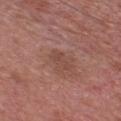{"biopsy_status": "not biopsied; imaged during a skin examination", "automated_metrics": {"vs_skin_darker_L": 6.0, "vs_skin_contrast_norm": 5.0, "border_irregularity_0_10": 5.0, "color_variation_0_10": 1.0, "peripheral_color_asymmetry": 0.0}, "lesion_size": {"long_diameter_mm_approx": 3.0}, "image": {"source": "total-body photography crop", "field_of_view_mm": 15}, "site": "chest", "lighting": "white-light", "patient": {"sex": "male", "age_approx": 70}}